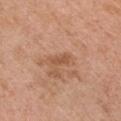| field | value |
|---|---|
| patient | male, approximately 70 years of age |
| TBP lesion metrics | internal color variation of about 0 on a 0–10 scale and peripheral color asymmetry of about 0 |
| imaging modality | ~15 mm crop, total-body skin-cancer survey |
| anatomic site | the right upper arm |
| tile lighting | white-light illumination |
| size | about 2.5 mm |